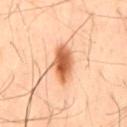Findings:
- workup · catalogued during a skin exam; not biopsied
- image source · total-body-photography crop, ~15 mm field of view
- TBP lesion metrics · about 17 CIELAB-L* units darker than the surrounding skin; a border-irregularity rating of about 2/10, a within-lesion color-variation index near 6/10, and peripheral color asymmetry of about 2
- subject · male, approximately 45 years of age
- site · the back